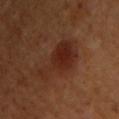Part of a total-body skin-imaging series; this lesion was reviewed on a skin check and was not flagged for biopsy.
From the chest.
A lesion tile, about 15 mm wide, cut from a 3D total-body photograph.
The subject is a male in their 50s.